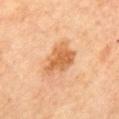| key | value |
|---|---|
| biopsy status | total-body-photography surveillance lesion; no biopsy |
| automated metrics | a footprint of about 11 mm², an outline eccentricity of about 0.8 (0 = round, 1 = elongated), and a symmetry-axis asymmetry near 0.35; a detector confidence of about 100 out of 100 that the crop contains a lesion |
| subject | female, approximately 70 years of age |
| image source | 15 mm crop, total-body photography |
| site | the mid back |
| lighting | cross-polarized |